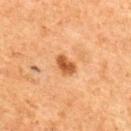  biopsy_status: not biopsied; imaged during a skin examination
  lesion_size:
    long_diameter_mm_approx: 2.5
  image:
    source: total-body photography crop
    field_of_view_mm: 15
  site: upper back
  automated_metrics:
    eccentricity: 0.75
    border_irregularity_0_10: 2.0
    color_variation_0_10: 2.0
    peripheral_color_asymmetry: 0.5
    nevus_likeness_0_100: 95
    lesion_detection_confidence_0_100: 100
  lighting: cross-polarized
  patient:
    sex: female
    age_approx: 60